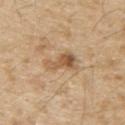| feature | finding |
|---|---|
| biopsy status | imaged on a skin check; not biopsied |
| diameter | ≈4 mm |
| patient | male, about 70 years old |
| imaging modality | ~15 mm crop, total-body skin-cancer survey |
| site | the upper back |